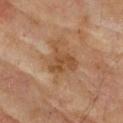The lesion was tiled from a total-body skin photograph and was not biopsied. About 4 mm across. A 15 mm crop from a total-body photograph taken for skin-cancer surveillance. Automated tile analysis of the lesion measured an area of roughly 9 mm² and an eccentricity of roughly 0.45. It also reported a mean CIELAB color near L≈40 a*≈17 b*≈30, about 7 CIELAB-L* units darker than the surrounding skin, and a lesion-to-skin contrast of about 6.5 (normalized; higher = more distinct). And it measured a classifier nevus-likeness of about 0/100 and lesion-presence confidence of about 100/100. The subject is a male aged 73 to 77. This is a cross-polarized tile. The lesion is on the upper back.